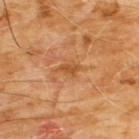Q: Was a biopsy performed?
A: total-body-photography surveillance lesion; no biopsy
Q: What is the lesion's diameter?
A: ~2.5 mm (longest diameter)
Q: What is the anatomic site?
A: the chest
Q: Who is the patient?
A: male, in their 60s
Q: How was this image acquired?
A: ~15 mm crop, total-body skin-cancer survey